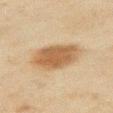biopsy status: no biopsy performed (imaged during a skin exam) | tile lighting: cross-polarized | body site: the left upper arm | patient: male, aged 43 to 47 | acquisition: ~15 mm crop, total-body skin-cancer survey | image-analysis metrics: a lesion-detection confidence of about 100/100.